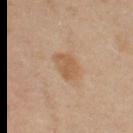notes=catalogued during a skin exam; not biopsied
size=≈3.5 mm
tile lighting=cross-polarized
patient=female, aged approximately 50
acquisition=~15 mm tile from a whole-body skin photo
body site=the left upper arm